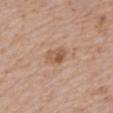Captured during whole-body skin photography for melanoma surveillance; the lesion was not biopsied. Captured under white-light illumination. The lesion's longest dimension is about 3 mm. Automated tile analysis of the lesion measured a classifier nevus-likeness of about 40/100. A female subject aged 73–77. The lesion is located on the mid back. This image is a 15 mm lesion crop taken from a total-body photograph.